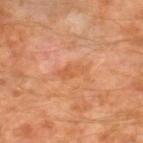Assessment: Captured during whole-body skin photography for melanoma surveillance; the lesion was not biopsied. Image and clinical context: From the right lower leg. A region of skin cropped from a whole-body photographic capture, roughly 15 mm wide. The lesion's longest dimension is about 3.5 mm. The subject is a male about 60 years old.